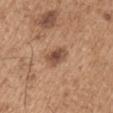{"biopsy_status": "not biopsied; imaged during a skin examination", "patient": {"sex": "male", "age_approx": 65}, "lesion_size": {"long_diameter_mm_approx": 3.0}, "site": "arm", "image": {"source": "total-body photography crop", "field_of_view_mm": 15}}